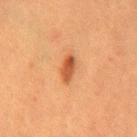<lesion>
<biopsy_status>not biopsied; imaged during a skin examination</biopsy_status>
<lesion_size>
  <long_diameter_mm_approx>3.0</long_diameter_mm_approx>
</lesion_size>
<patient>
  <sex>male</sex>
  <age_approx>50</age_approx>
</patient>
<site>mid back</site>
<automated_metrics>
  <border_irregularity_0_10>2.5</border_irregularity_0_10>
  <peripheral_color_asymmetry>1.5</peripheral_color_asymmetry>
</automated_metrics>
<image>
  <source>total-body photography crop</source>
  <field_of_view_mm>15</field_of_view_mm>
</image>
</lesion>